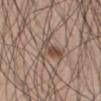Impression:
The lesion was photographed on a routine skin check and not biopsied; there is no pathology result.
Acquisition and patient details:
A region of skin cropped from a whole-body photographic capture, roughly 15 mm wide. On the abdomen. The patient is a male in their mid-60s.